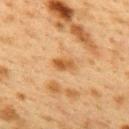Impression: This lesion was catalogued during total-body skin photography and was not selected for biopsy. Context: From the upper back. The tile uses cross-polarized illumination. Longest diameter approximately 2.5 mm. A roughly 15 mm field-of-view crop from a total-body skin photograph. A female patient, about 40 years old.